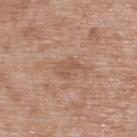Captured during whole-body skin photography for melanoma surveillance; the lesion was not biopsied. The lesion is located on the upper back. The patient is a male in their 50s. Captured under white-light illumination. A close-up tile cropped from a whole-body skin photograph, about 15 mm across.